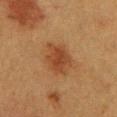{"site": "front of the torso", "automated_metrics": {"area_mm2_approx": 9.0, "eccentricity": 0.7, "shape_asymmetry": 0.25, "cielab_L": 35, "cielab_a": 20, "cielab_b": 31, "vs_skin_darker_L": 8.0, "vs_skin_contrast_norm": 7.5}, "patient": {"sex": "female", "age_approx": 40}, "image": {"source": "total-body photography crop", "field_of_view_mm": 15}}